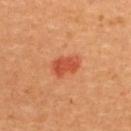| field | value |
|---|---|
| notes | catalogued during a skin exam; not biopsied |
| patient | female, aged around 30 |
| anatomic site | the upper back |
| imaging modality | total-body-photography crop, ~15 mm field of view |